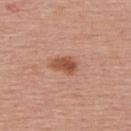Part of a total-body skin-imaging series; this lesion was reviewed on a skin check and was not flagged for biopsy. The recorded lesion diameter is about 3.5 mm. A 15 mm close-up extracted from a 3D total-body photography capture. The lesion is on the upper back. The patient is a female in their mid- to late 50s. The tile uses white-light illumination.